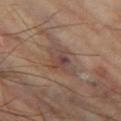Imaged with cross-polarized lighting. The lesion's longest dimension is about 4.5 mm. A male subject, in their mid-60s. Cropped from a whole-body photographic skin survey; the tile spans about 15 mm. The lesion is located on the left thigh.